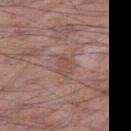Captured during whole-body skin photography for melanoma surveillance; the lesion was not biopsied. From the leg. A male subject aged 53–57. A region of skin cropped from a whole-body photographic capture, roughly 15 mm wide. About 3 mm across. Automated image analysis of the tile measured roughly 7 lightness units darker than nearby skin and a normalized border contrast of about 5.5.